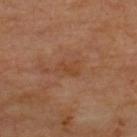This lesion was catalogued during total-body skin photography and was not selected for biopsy.
The recorded lesion diameter is about 2.5 mm.
An algorithmic analysis of the crop reported a shape-asymmetry score of about 0.35 (0 = symmetric). The software also gave an average lesion color of about L≈43 a*≈22 b*≈33 (CIELAB), about 5 CIELAB-L* units darker than the surrounding skin, and a lesion-to-skin contrast of about 5.5 (normalized; higher = more distinct). The software also gave a classifier nevus-likeness of about 0/100 and lesion-presence confidence of about 100/100.
A 15 mm crop from a total-body photograph taken for skin-cancer surveillance.
This is a cross-polarized tile.
The lesion is on the upper back.
The patient is roughly 65 years of age.